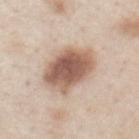– follow-up: no biopsy performed (imaged during a skin exam)
– diameter: ~6.5 mm (longest diameter)
– image: ~15 mm crop, total-body skin-cancer survey
– tile lighting: white-light
– patient: male, aged approximately 60
– anatomic site: the chest